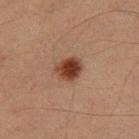Q: Was this lesion biopsied?
A: catalogued during a skin exam; not biopsied
Q: Who is the patient?
A: male, in their 40s
Q: Illumination type?
A: cross-polarized illumination
Q: How was this image acquired?
A: total-body-photography crop, ~15 mm field of view
Q: Automated lesion metrics?
A: a lesion area of about 6 mm², an eccentricity of roughly 0.5, and a symmetry-axis asymmetry near 0.2; a lesion color around L≈31 a*≈20 b*≈25 in CIELAB and roughly 13 lightness units darker than nearby skin; internal color variation of about 3.5 on a 0–10 scale and radial color variation of about 1; a classifier nevus-likeness of about 100/100
Q: Lesion size?
A: ~3 mm (longest diameter)
Q: Lesion location?
A: the right thigh